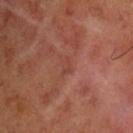Captured during whole-body skin photography for melanoma surveillance; the lesion was not biopsied.
Located on the left upper arm.
The patient is a male approximately 60 years of age.
Automated tile analysis of the lesion measured an eccentricity of roughly 0.8 and a symmetry-axis asymmetry near 0.35. It also reported a lesion color around L≈31 a*≈20 b*≈21 in CIELAB and a lesion-to-skin contrast of about 4 (normalized; higher = more distinct).
Longest diameter approximately 2 mm.
Cropped from a total-body skin-imaging series; the visible field is about 15 mm.